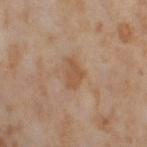notes: catalogued during a skin exam; not biopsied | tile lighting: cross-polarized | image source: ~15 mm tile from a whole-body skin photo | lesion size: ≈3.5 mm | site: the right thigh | subject: female, approximately 55 years of age.